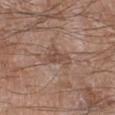The lesion was photographed on a routine skin check and not biopsied; there is no pathology result.
A region of skin cropped from a whole-body photographic capture, roughly 15 mm wide.
A male patient approximately 60 years of age.
The lesion is on the right lower leg.